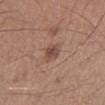Imaged during a routine full-body skin examination; the lesion was not biopsied and no histopathology is available.